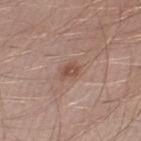The lesion was tiled from a total-body skin photograph and was not biopsied. The total-body-photography lesion software estimated an area of roughly 3.5 mm² and a shape eccentricity near 0.75. The software also gave a border-irregularity rating of about 3/10 and a within-lesion color-variation index near 2.5/10. The analysis additionally found a nevus-likeness score of about 35/100 and lesion-presence confidence of about 100/100. A male subject approximately 30 years of age. The lesion is located on the left lower leg. A lesion tile, about 15 mm wide, cut from a 3D total-body photograph. Measured at roughly 2.5 mm in maximum diameter. The tile uses white-light illumination.